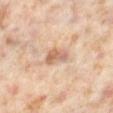{"biopsy_status": "not biopsied; imaged during a skin examination", "site": "leg", "patient": {"sex": "female", "age_approx": 50}, "automated_metrics": {"area_mm2_approx": 5.5, "shape_asymmetry": 0.3, "border_irregularity_0_10": 3.0, "peripheral_color_asymmetry": 2.0}, "image": {"source": "total-body photography crop", "field_of_view_mm": 15}, "lesion_size": {"long_diameter_mm_approx": 3.0}}